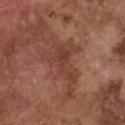notes: no biopsy performed (imaged during a skin exam)
image-analysis metrics: a footprint of about 18 mm²; an average lesion color of about L≈41 a*≈23 b*≈27 (CIELAB) and a lesion–skin lightness drop of about 7; a classifier nevus-likeness of about 0/100 and a detector confidence of about 85 out of 100 that the crop contains a lesion
tile lighting: white-light illumination
acquisition: total-body-photography crop, ~15 mm field of view
anatomic site: the chest
subject: male, about 75 years old